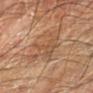Impression:
No biopsy was performed on this lesion — it was imaged during a full skin examination and was not determined to be concerning.
Acquisition and patient details:
A male subject, aged 68 to 72. A 15 mm close-up extracted from a 3D total-body photography capture. Captured under cross-polarized illumination. On the left forearm.